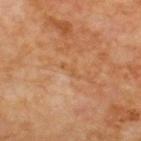The lesion was tiled from a total-body skin photograph and was not biopsied.
A male patient, roughly 70 years of age.
The lesion-visualizer software estimated a lesion area of about 2 mm², an outline eccentricity of about 0.95 (0 = round, 1 = elongated), and a symmetry-axis asymmetry near 0.5. The analysis additionally found a lesion color around L≈54 a*≈22 b*≈39 in CIELAB and a normalized lesion–skin contrast near 4.5. The analysis additionally found a detector confidence of about 90 out of 100 that the crop contains a lesion.
About 2.5 mm across.
A 15 mm crop from a total-body photograph taken for skin-cancer surveillance.
On the upper back.